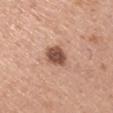notes: imaged on a skin check; not biopsied
image: ~15 mm tile from a whole-body skin photo
subject: male, about 55 years old
location: the left upper arm